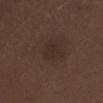The lesion was photographed on a routine skin check and not biopsied; there is no pathology result.
Located on the right lower leg.
A male subject, aged 68 to 72.
About 3 mm across.
This is a white-light tile.
A region of skin cropped from a whole-body photographic capture, roughly 15 mm wide.